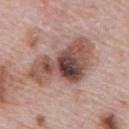patient: male, aged approximately 70 | illumination: white-light illumination | anatomic site: the abdomen | TBP lesion metrics: an outline eccentricity of about 0.85 (0 = round, 1 = elongated) and a shape-asymmetry score of about 0.4 (0 = symmetric); a lesion color around L≈50 a*≈19 b*≈25 in CIELAB, about 15 CIELAB-L* units darker than the surrounding skin, and a normalized border contrast of about 10.5; a classifier nevus-likeness of about 10/100 and a detector confidence of about 100 out of 100 that the crop contains a lesion | image: ~15 mm crop, total-body skin-cancer survey | lesion size: about 9 mm.